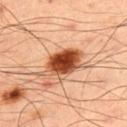* follow-up: no biopsy performed (imaged during a skin exam)
* TBP lesion metrics: a footprint of about 14 mm², an eccentricity of roughly 0.8, and a shape-asymmetry score of about 0.25 (0 = symmetric); a lesion-detection confidence of about 100/100
* acquisition: ~15 mm crop, total-body skin-cancer survey
* diameter: ~6 mm (longest diameter)
* location: the upper back
* tile lighting: cross-polarized illumination
* subject: male, aged approximately 50